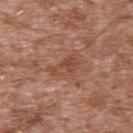This lesion was catalogued during total-body skin photography and was not selected for biopsy. From the upper back. This image is a 15 mm lesion crop taken from a total-body photograph. A male subject, in their mid-40s. Measured at roughly 4 mm in maximum diameter.